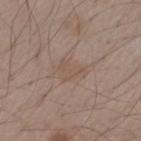The lesion was photographed on a routine skin check and not biopsied; there is no pathology result. A male patient, aged approximately 55. Automated image analysis of the tile measured an average lesion color of about L≈52 a*≈16 b*≈26 (CIELAB). It also reported a border-irregularity index near 6.5/10. The analysis additionally found a nevus-likeness score of about 0/100 and a detector confidence of about 100 out of 100 that the crop contains a lesion. The tile uses white-light illumination. A region of skin cropped from a whole-body photographic capture, roughly 15 mm wide. The lesion is on the right forearm.